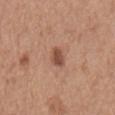Notes:
– biopsy status — total-body-photography surveillance lesion; no biopsy
– lesion diameter — about 3 mm
– anatomic site — the mid back
– tile lighting — white-light illumination
– patient — male, roughly 65 years of age
– imaging modality — ~15 mm tile from a whole-body skin photo
– image-analysis metrics — an area of roughly 4 mm² and an eccentricity of roughly 0.7